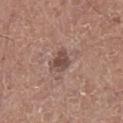Assessment: This lesion was catalogued during total-body skin photography and was not selected for biopsy. Clinical summary: This image is a 15 mm lesion crop taken from a total-body photograph. The lesion is located on the left lower leg. Imaged with white-light lighting. A female subject aged 48 to 52. The recorded lesion diameter is about 2.5 mm.